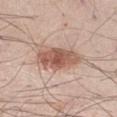| field | value |
|---|---|
| biopsy status | no biopsy performed (imaged during a skin exam) |
| image source | ~15 mm crop, total-body skin-cancer survey |
| tile lighting | white-light |
| anatomic site | the leg |
| subject | male, aged 43–47 |